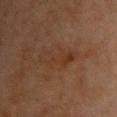Recorded during total-body skin imaging; not selected for excision or biopsy. This image is a 15 mm lesion crop taken from a total-body photograph. A female subject aged around 70. On the front of the torso.